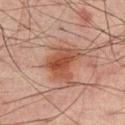This lesion was catalogued during total-body skin photography and was not selected for biopsy. This is a cross-polarized tile. The lesion is located on the mid back. A 15 mm close-up tile from a total-body photography series done for melanoma screening. A male patient, in their mid- to late 60s. About 4.5 mm across.